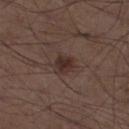Clinical impression:
This lesion was catalogued during total-body skin photography and was not selected for biopsy.
Background:
A roughly 15 mm field-of-view crop from a total-body skin photograph. The recorded lesion diameter is about 3 mm. A male patient, in their 50s. The lesion is on the left thigh. Captured under white-light illumination.